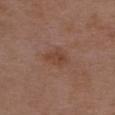The lesion was tiled from a total-body skin photograph and was not biopsied. A region of skin cropped from a whole-body photographic capture, roughly 15 mm wide. A female patient aged 28 to 32. The lesion-visualizer software estimated an area of roughly 4.5 mm² and a symmetry-axis asymmetry near 0.25. It also reported a lesion color around L≈43 a*≈21 b*≈28 in CIELAB, a lesion–skin lightness drop of about 7, and a lesion-to-skin contrast of about 6 (normalized; higher = more distinct). On the back. The recorded lesion diameter is about 3 mm. This is a white-light tile.